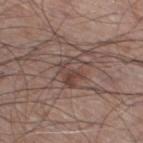workup = catalogued during a skin exam; not biopsied | diameter = ~3 mm (longest diameter) | illumination = white-light illumination | patient = male, aged approximately 60 | image source = 15 mm crop, total-body photography | body site = the right thigh.